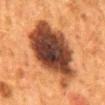{"biopsy_status": "not biopsied; imaged during a skin examination", "automated_metrics": {"area_mm2_approx": 40.0, "eccentricity": 0.8, "shape_asymmetry": 0.2, "border_irregularity_0_10": 3.0, "peripheral_color_asymmetry": 2.0, "nevus_likeness_0_100": 45, "lesion_detection_confidence_0_100": 100}, "lighting": "cross-polarized", "patient": {"sex": "female", "age_approx": 50}, "image": {"source": "total-body photography crop", "field_of_view_mm": 15}, "site": "mid back", "lesion_size": {"long_diameter_mm_approx": 10.0}}